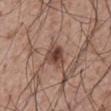| feature | finding |
|---|---|
| subject | male, aged approximately 60 |
| location | the abdomen |
| imaging modality | 15 mm crop, total-body photography |